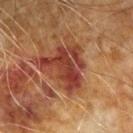notes: imaged on a skin check; not biopsied | image source: total-body-photography crop, ~15 mm field of view | illumination: cross-polarized illumination | patient: male, aged 68–72 | automated metrics: a lesion area of about 15 mm², a shape eccentricity near 0.65, and a symmetry-axis asymmetry near 0.5; a lesion-to-skin contrast of about 9.5 (normalized; higher = more distinct); internal color variation of about 4.5 on a 0–10 scale and peripheral color asymmetry of about 1.5; a lesion-detection confidence of about 100/100 | location: the left upper arm.